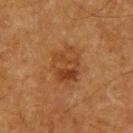Part of a total-body skin-imaging series; this lesion was reviewed on a skin check and was not flagged for biopsy.
A region of skin cropped from a whole-body photographic capture, roughly 15 mm wide.
A male subject, in their mid-60s.
This is a cross-polarized tile.
From the upper back.
Automated tile analysis of the lesion measured a border-irregularity rating of about 2.5/10, a within-lesion color-variation index near 5/10, and peripheral color asymmetry of about 2. And it measured an automated nevus-likeness rating near 50 out of 100 and a lesion-detection confidence of about 100/100.